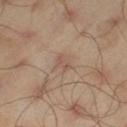Recorded during total-body skin imaging; not selected for excision or biopsy. The lesion is on the left thigh. A roughly 15 mm field-of-view crop from a total-body skin photograph. A male subject aged approximately 45. The recorded lesion diameter is about 2.5 mm. The tile uses cross-polarized illumination.